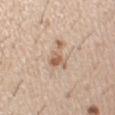Clinical impression: The lesion was photographed on a routine skin check and not biopsied; there is no pathology result. Context: The total-body-photography lesion software estimated a mean CIELAB color near L≈61 a*≈17 b*≈30, roughly 10 lightness units darker than nearby skin, and a normalized lesion–skin contrast near 7. It also reported a border-irregularity rating of about 7/10 and a peripheral color-asymmetry measure near 1.5. And it measured a nevus-likeness score of about 5/100. Captured under white-light illumination. Longest diameter approximately 4.5 mm. Located on the right upper arm. A male patient, about 60 years old. This image is a 15 mm lesion crop taken from a total-body photograph.